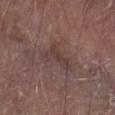- notes: catalogued during a skin exam; not biopsied
- imaging modality: ~15 mm tile from a whole-body skin photo
- patient: male, approximately 65 years of age
- site: the right forearm